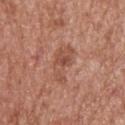Q: How was the tile lit?
A: white-light
Q: Lesion size?
A: ~5 mm (longest diameter)
Q: What kind of image is this?
A: ~15 mm tile from a whole-body skin photo
Q: Who is the patient?
A: male, aged 63 to 67
Q: Where on the body is the lesion?
A: the upper back